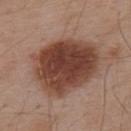{
  "patient": {
    "sex": "male",
    "age_approx": 55
  },
  "image": {
    "source": "total-body photography crop",
    "field_of_view_mm": 15
  },
  "automated_metrics": {
    "area_mm2_approx": 39.0,
    "eccentricity": 0.65,
    "shape_asymmetry": 0.15,
    "vs_skin_darker_L": 15.0,
    "vs_skin_contrast_norm": 11.5,
    "nevus_likeness_0_100": 95
  },
  "site": "back",
  "lighting": "white-light",
  "lesion_size": {
    "long_diameter_mm_approx": 8.0
  }
}